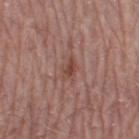The lesion was photographed on a routine skin check and not biopsied; there is no pathology result.
The tile uses white-light illumination.
The subject is a male roughly 55 years of age.
A lesion tile, about 15 mm wide, cut from a 3D total-body photograph.
The lesion's longest dimension is about 3 mm.
Located on the right thigh.